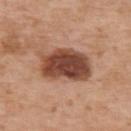Impression: The lesion was photographed on a routine skin check and not biopsied; there is no pathology result. Image and clinical context: Measured at roughly 7 mm in maximum diameter. A region of skin cropped from a whole-body photographic capture, roughly 15 mm wide. An algorithmic analysis of the crop reported a mean CIELAB color near L≈46 a*≈23 b*≈29, about 17 CIELAB-L* units darker than the surrounding skin, and a normalized border contrast of about 12. The software also gave a classifier nevus-likeness of about 95/100. A male patient, aged 58 to 62. Captured under white-light illumination. On the upper back.